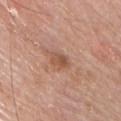<record>
<biopsy_status>not biopsied; imaged during a skin examination</biopsy_status>
<automated_metrics>
  <area_mm2_approx>4.0</area_mm2_approx>
  <shape_asymmetry>0.25</shape_asymmetry>
  <cielab_L>53</cielab_L>
  <cielab_a>22</cielab_a>
  <cielab_b>30</cielab_b>
  <vs_skin_contrast_norm>6.5</vs_skin_contrast_norm>
</automated_metrics>
<patient>
  <sex>male</sex>
  <age_approx>65</age_approx>
</patient>
<image>
  <source>total-body photography crop</source>
  <field_of_view_mm>15</field_of_view_mm>
</image>
<lighting>white-light</lighting>
<site>chest</site>
</record>